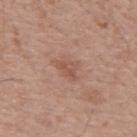{"lesion_size": {"long_diameter_mm_approx": 3.5}, "site": "mid back", "lighting": "white-light", "automated_metrics": {"eccentricity": 0.7, "cielab_L": 54, "cielab_a": 21, "cielab_b": 28, "vs_skin_darker_L": 7.0, "vs_skin_contrast_norm": 5.0, "border_irregularity_0_10": 4.5, "peripheral_color_asymmetry": 1.0}, "image": {"source": "total-body photography crop", "field_of_view_mm": 15}, "patient": {"sex": "male", "age_approx": 55}}